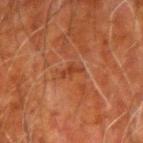Imaged during a routine full-body skin examination; the lesion was not biopsied and no histopathology is available. Longest diameter approximately 2.5 mm. A male patient, in their 80s. The lesion is located on the left upper arm. The total-body-photography lesion software estimated an outline eccentricity of about 0.95 (0 = round, 1 = elongated) and a symmetry-axis asymmetry near 0.5. It also reported a lesion color around L≈32 a*≈25 b*≈30 in CIELAB. It also reported a border-irregularity index near 5.5/10 and a peripheral color-asymmetry measure near 0. This image is a 15 mm lesion crop taken from a total-body photograph.